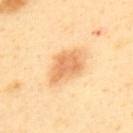Captured during whole-body skin photography for melanoma surveillance; the lesion was not biopsied.
The patient is a male in their 40s.
A 15 mm close-up tile from a total-body photography series done for melanoma screening.
Captured under cross-polarized illumination.
Longest diameter approximately 5 mm.
The lesion is located on the upper back.